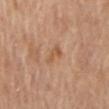The tile uses cross-polarized illumination. A male subject aged 68 to 72. About 3 mm across. A 15 mm close-up extracted from a 3D total-body photography capture. From the mid back.